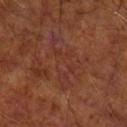Impression: Part of a total-body skin-imaging series; this lesion was reviewed on a skin check and was not flagged for biopsy. Background: The patient is a male in their mid- to late 60s. About 6.5 mm across. Imaged with cross-polarized lighting. A region of skin cropped from a whole-body photographic capture, roughly 15 mm wide. The lesion is located on the arm.